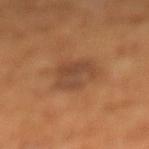  automated_metrics:
    vs_skin_contrast_norm: 6.5
    border_irregularity_0_10: 4.0
    nevus_likeness_0_100: 5
    lesion_detection_confidence_0_100: 100
  site: leg
  patient:
    sex: female
    age_approx: 65
  lighting: cross-polarized
  lesion_size:
    long_diameter_mm_approx: 4.0
  image:
    source: total-body photography crop
    field_of_view_mm: 15